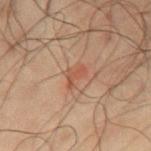Part of a total-body skin-imaging series; this lesion was reviewed on a skin check and was not flagged for biopsy. From the chest. Captured under cross-polarized illumination. A 15 mm close-up tile from a total-body photography series done for melanoma screening. A male patient, in their 50s. Longest diameter approximately 2.5 mm.